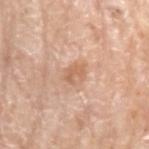{"biopsy_status": "not biopsied; imaged during a skin examination", "site": "left upper arm", "lighting": "white-light", "patient": {"sex": "female", "age_approx": 75}, "lesion_size": {"long_diameter_mm_approx": 3.0}, "image": {"source": "total-body photography crop", "field_of_view_mm": 15}, "automated_metrics": {"area_mm2_approx": 4.5, "eccentricity": 0.7, "cielab_L": 63, "cielab_a": 21, "cielab_b": 33, "vs_skin_contrast_norm": 6.0, "border_irregularity_0_10": 3.0, "color_variation_0_10": 3.5, "peripheral_color_asymmetry": 1.5}}